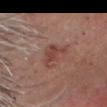Case summary:
– follow-up — total-body-photography surveillance lesion; no biopsy
– anatomic site — the head or neck
– acquisition — ~15 mm crop, total-body skin-cancer survey
– patient — male, aged 58 to 62
– lesion diameter — about 3 mm